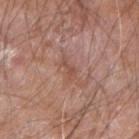biopsy status: no biopsy performed (imaged during a skin exam); illumination: white-light illumination; subject: male, approximately 60 years of age; image: ~15 mm crop, total-body skin-cancer survey; location: the right forearm.